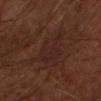follow-up: imaged on a skin check; not biopsied
lesion size: ≈6 mm
lighting: cross-polarized illumination
site: the right forearm
patient: male, approximately 65 years of age
image source: total-body-photography crop, ~15 mm field of view